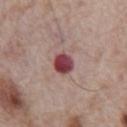{
  "image": {
    "source": "total-body photography crop",
    "field_of_view_mm": 15
  },
  "lesion_size": {
    "long_diameter_mm_approx": 3.0
  },
  "site": "front of the torso",
  "lighting": "white-light",
  "patient": {
    "sex": "male",
    "age_approx": 70
  }
}